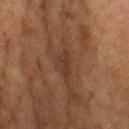follow-up: catalogued during a skin exam; not biopsied
image: ~15 mm crop, total-body skin-cancer survey
subject: male, aged around 45
body site: the head or neck